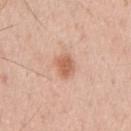{
  "biopsy_status": "not biopsied; imaged during a skin examination",
  "patient": {
    "sex": "male",
    "age_approx": 55
  },
  "lighting": "white-light",
  "image": {
    "source": "total-body photography crop",
    "field_of_view_mm": 15
  },
  "lesion_size": {
    "long_diameter_mm_approx": 3.0
  },
  "automated_metrics": {
    "area_mm2_approx": 5.5,
    "eccentricity": 0.7,
    "shape_asymmetry": 0.25,
    "cielab_L": 61,
    "cielab_a": 24,
    "cielab_b": 32,
    "nevus_likeness_0_100": 90
  },
  "site": "chest"
}